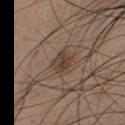Q: Is there a histopathology result?
A: total-body-photography surveillance lesion; no biopsy
Q: What is the imaging modality?
A: ~15 mm crop, total-body skin-cancer survey
Q: Patient demographics?
A: male, roughly 20 years of age
Q: How was the tile lit?
A: white-light
Q: Where on the body is the lesion?
A: the chest